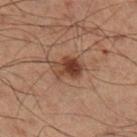Q: Is there a histopathology result?
A: no biopsy performed (imaged during a skin exam)
Q: What did automated image analysis measure?
A: a footprint of about 8.5 mm², an outline eccentricity of about 0.7 (0 = round, 1 = elongated), and a shape-asymmetry score of about 0.25 (0 = symmetric); a classifier nevus-likeness of about 95/100
Q: Patient demographics?
A: male, aged 58–62
Q: What is the lesion's diameter?
A: ~4 mm (longest diameter)
Q: Lesion location?
A: the right lower leg
Q: Illumination type?
A: cross-polarized illumination
Q: What kind of image is this?
A: total-body-photography crop, ~15 mm field of view